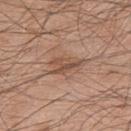biopsy status — no biopsy performed (imaged during a skin exam)
tile lighting — white-light illumination
acquisition — ~15 mm crop, total-body skin-cancer survey
body site — the upper back
patient — male, in their mid- to late 40s